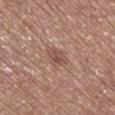Findings:
– follow-up — imaged on a skin check; not biopsied
– image — total-body-photography crop, ~15 mm field of view
– lesion size — about 3 mm
– lighting — white-light illumination
– anatomic site — the right lower leg
– subject — male, aged 73 to 77